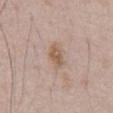No biopsy was performed on this lesion — it was imaged during a full skin examination and was not determined to be concerning.
Cropped from a whole-body photographic skin survey; the tile spans about 15 mm.
Located on the abdomen.
A male subject about 65 years old.